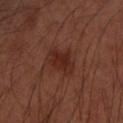{"lighting": "cross-polarized", "lesion_size": {"long_diameter_mm_approx": 4.0}, "site": "left arm", "patient": {"sex": "male", "age_approx": 50}, "image": {"source": "total-body photography crop", "field_of_view_mm": 15}, "automated_metrics": {"vs_skin_darker_L": 7.0, "vs_skin_contrast_norm": 7.0, "border_irregularity_0_10": 3.0, "color_variation_0_10": 2.0, "peripheral_color_asymmetry": 0.5, "nevus_likeness_0_100": 25, "lesion_detection_confidence_0_100": 100}}